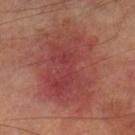The lesion was tiled from a total-body skin photograph and was not biopsied.